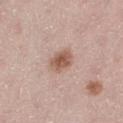Measured at roughly 3 mm in maximum diameter. Captured under white-light illumination. Cropped from a total-body skin-imaging series; the visible field is about 15 mm. Located on the left thigh. The patient is a female aged 38–42.